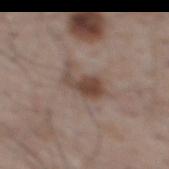Findings:
– workup · imaged on a skin check; not biopsied
– patient · male, in their mid-60s
– image · ~15 mm tile from a whole-body skin photo
– site · the upper back